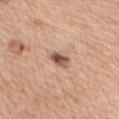{"biopsy_status": "not biopsied; imaged during a skin examination", "image": {"source": "total-body photography crop", "field_of_view_mm": 15}, "patient": {"sex": "female", "age_approx": 55}, "site": "right upper arm"}